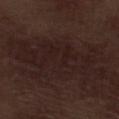This lesion was catalogued during total-body skin photography and was not selected for biopsy. Approximately 11.5 mm at its widest. A roughly 15 mm field-of-view crop from a total-body skin photograph. A male patient approximately 70 years of age. The total-body-photography lesion software estimated an eccentricity of roughly 0.9 and two-axis asymmetry of about 0.35. The analysis additionally found a lesion–skin lightness drop of about 5 and a normalized border contrast of about 6.5. The software also gave a border-irregularity index near 7.5/10 and a color-variation rating of about 2.5/10. The analysis additionally found lesion-presence confidence of about 55/100. Located on the left lower leg.